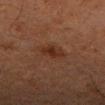Part of a total-body skin-imaging series; this lesion was reviewed on a skin check and was not flagged for biopsy. The lesion is on the right forearm. A close-up tile cropped from a whole-body skin photograph, about 15 mm across. Approximately 3 mm at its widest. This is a cross-polarized tile. A female patient aged 58 to 62. An algorithmic analysis of the crop reported a nevus-likeness score of about 85/100.